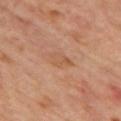Recorded during total-body skin imaging; not selected for excision or biopsy. Automated tile analysis of the lesion measured an area of roughly 4 mm² and two-axis asymmetry of about 0.4. The software also gave lesion-presence confidence of about 100/100. The tile uses cross-polarized illumination. The lesion is located on the mid back. The subject is a male roughly 60 years of age. About 3 mm across. A 15 mm close-up extracted from a 3D total-body photography capture.